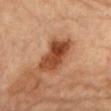Findings:
* biopsy status: imaged on a skin check; not biopsied
* image: ~15 mm crop, total-body skin-cancer survey
* site: the front of the torso
* lesion size: ≈5.5 mm
* patient: female, in their 60s
* illumination: cross-polarized illumination